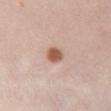Q: Was a biopsy performed?
A: catalogued during a skin exam; not biopsied
Q: How was this image acquired?
A: 15 mm crop, total-body photography
Q: Automated lesion metrics?
A: a border-irregularity index near 1.5/10, a within-lesion color-variation index near 3/10, and a peripheral color-asymmetry measure near 1; a lesion-detection confidence of about 100/100
Q: How was the tile lit?
A: white-light
Q: Lesion location?
A: the chest
Q: What are the patient's age and sex?
A: female, in their 50s
Q: How large is the lesion?
A: ≈2.5 mm